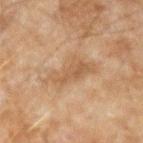The lesion was photographed on a routine skin check and not biopsied; there is no pathology result. A male patient approximately 45 years of age. This is a cross-polarized tile. This image is a 15 mm lesion crop taken from a total-body photograph. On the left forearm.